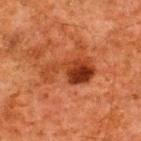Q: Is there a histopathology result?
A: no biopsy performed (imaged during a skin exam)
Q: Patient demographics?
A: male, about 60 years old
Q: Lesion location?
A: the upper back
Q: What is the imaging modality?
A: total-body-photography crop, ~15 mm field of view